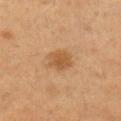Findings:
* workup: catalogued during a skin exam; not biopsied
* tile lighting: cross-polarized
* image source: ~15 mm crop, total-body skin-cancer survey
* patient: male, in their mid- to late 50s
* site: the right upper arm
* lesion size: ≈3 mm
* automated metrics: a border-irregularity index near 2/10, a within-lesion color-variation index near 2/10, and a peripheral color-asymmetry measure near 1